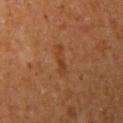Notes:
– workup · total-body-photography surveillance lesion; no biopsy
– image · ~15 mm crop, total-body skin-cancer survey
– site · the right upper arm
– TBP lesion metrics · a footprint of about 3.5 mm², an eccentricity of roughly 0.95, and a symmetry-axis asymmetry near 0.35; a border-irregularity index near 4.5/10, internal color variation of about 1 on a 0–10 scale, and radial color variation of about 0; a nevus-likeness score of about 0/100
– tile lighting · cross-polarized
– lesion size · ≈3.5 mm
– patient · female